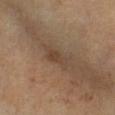The tile uses cross-polarized illumination.
The subject is a female about 70 years old.
An algorithmic analysis of the crop reported a footprint of about 5 mm² and an eccentricity of roughly 0.9. The analysis additionally found a lesion–skin lightness drop of about 6 and a normalized border contrast of about 5.5. It also reported a border-irregularity index near 3.5/10, internal color variation of about 3 on a 0–10 scale, and a peripheral color-asymmetry measure near 1. The software also gave a nevus-likeness score of about 5/100 and a lesion-detection confidence of about 100/100.
Located on the right lower leg.
This image is a 15 mm lesion crop taken from a total-body photograph.
The recorded lesion diameter is about 3.5 mm.